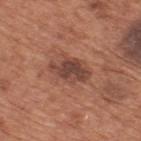Q: Was a biopsy performed?
A: no biopsy performed (imaged during a skin exam)
Q: How was the tile lit?
A: white-light illumination
Q: What is the imaging modality?
A: total-body-photography crop, ~15 mm field of view
Q: Lesion location?
A: the upper back
Q: What is the lesion's diameter?
A: ~4.5 mm (longest diameter)
Q: Patient demographics?
A: male, aged 63–67
Q: Automated lesion metrics?
A: an average lesion color of about L≈44 a*≈23 b*≈27 (CIELAB), a lesion–skin lightness drop of about 11, and a normalized lesion–skin contrast near 8.5; a nevus-likeness score of about 50/100